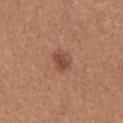Q: Was a biopsy performed?
A: catalogued during a skin exam; not biopsied
Q: Where on the body is the lesion?
A: the upper back
Q: What kind of image is this?
A: ~15 mm tile from a whole-body skin photo
Q: What are the patient's age and sex?
A: female, aged 38 to 42